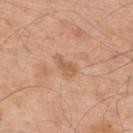{
  "biopsy_status": "not biopsied; imaged during a skin examination",
  "lesion_size": {
    "long_diameter_mm_approx": 3.0
  },
  "lighting": "white-light",
  "patient": {
    "sex": "male",
    "age_approx": 55
  },
  "image": {
    "source": "total-body photography crop",
    "field_of_view_mm": 15
  },
  "site": "upper back",
  "automated_metrics": {
    "cielab_L": 59,
    "cielab_a": 21,
    "cielab_b": 35,
    "vs_skin_darker_L": 8.0,
    "vs_skin_contrast_norm": 6.0,
    "border_irregularity_0_10": 3.5,
    "color_variation_0_10": 1.5,
    "peripheral_color_asymmetry": 0.5,
    "lesion_detection_confidence_0_100": 100
  }
}